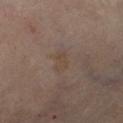Clinical impression: Part of a total-body skin-imaging series; this lesion was reviewed on a skin check and was not flagged for biopsy. Image and clinical context: Automated image analysis of the tile measured a footprint of about 4 mm² and an eccentricity of roughly 0.9. And it measured roughly 4 lightness units darker than nearby skin and a normalized lesion–skin contrast near 5. It also reported a classifier nevus-likeness of about 0/100. Cropped from a total-body skin-imaging series; the visible field is about 15 mm. On the right thigh. Captured under cross-polarized illumination. The subject is a female aged around 60.